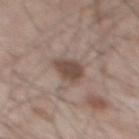A male patient aged around 50.
The total-body-photography lesion software estimated a lesion area of about 7 mm², an outline eccentricity of about 0.6 (0 = round, 1 = elongated), and a symmetry-axis asymmetry near 0.3. The software also gave a lesion color around L≈47 a*≈15 b*≈23 in CIELAB, about 12 CIELAB-L* units darker than the surrounding skin, and a lesion-to-skin contrast of about 8.5 (normalized; higher = more distinct). And it measured a border-irregularity index near 2.5/10, internal color variation of about 3 on a 0–10 scale, and peripheral color asymmetry of about 1. The software also gave a classifier nevus-likeness of about 65/100.
A 15 mm crop from a total-body photograph taken for skin-cancer surveillance.
Longest diameter approximately 3.5 mm.
Captured under white-light illumination.
The lesion is located on the mid back.